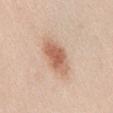The lesion was tiled from a total-body skin photograph and was not biopsied. A female subject, aged 38–42. The tile uses white-light illumination. The lesion is located on the abdomen. A lesion tile, about 15 mm wide, cut from a 3D total-body photograph.